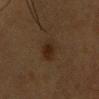The lesion was tiled from a total-body skin photograph and was not biopsied. Imaged with cross-polarized lighting. A region of skin cropped from a whole-body photographic capture, roughly 15 mm wide. Automated tile analysis of the lesion measured a border-irregularity rating of about 2/10, internal color variation of about 4 on a 0–10 scale, and a peripheral color-asymmetry measure near 1. A female subject aged 43 to 47. The lesion is located on the head or neck.